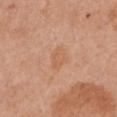- follow-up — catalogued during a skin exam; not biopsied
- location — the chest
- illumination — white-light illumination
- subject — female, about 55 years old
- acquisition — 15 mm crop, total-body photography